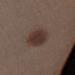Notes:
- notes: no biopsy performed (imaged during a skin exam)
- image source: 15 mm crop, total-body photography
- size: ~4.5 mm (longest diameter)
- location: the leg
- subject: female, aged approximately 40
- illumination: white-light illumination